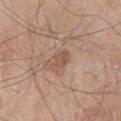Q: What kind of image is this?
A: ~15 mm tile from a whole-body skin photo
Q: What is the anatomic site?
A: the right thigh
Q: Patient demographics?
A: male, approximately 80 years of age